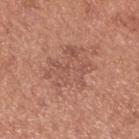Impression: Recorded during total-body skin imaging; not selected for excision or biopsy. Context: Located on the upper back. Cropped from a total-body skin-imaging series; the visible field is about 15 mm. Imaged with white-light lighting. The recorded lesion diameter is about 5.5 mm. A male patient aged 53 to 57.